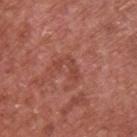{"biopsy_status": "not biopsied; imaged during a skin examination", "patient": {"sex": "male", "age_approx": 65}, "automated_metrics": {"area_mm2_approx": 4.5, "eccentricity": 0.8, "shape_asymmetry": 0.6, "nevus_likeness_0_100": 0, "lesion_detection_confidence_0_100": 100}, "site": "upper back", "image": {"source": "total-body photography crop", "field_of_view_mm": 15}}